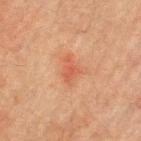Assessment:
This lesion was catalogued during total-body skin photography and was not selected for biopsy.
Image and clinical context:
The lesion's longest dimension is about 3 mm. Located on the left upper arm. An algorithmic analysis of the crop reported a footprint of about 4.5 mm². It also reported an average lesion color of about L≈48 a*≈26 b*≈31 (CIELAB), a lesion–skin lightness drop of about 7, and a normalized border contrast of about 5.5. And it measured a border-irregularity rating of about 4/10 and a within-lesion color-variation index near 1.5/10. It also reported a nevus-likeness score of about 5/100. A male patient, in their mid- to late 70s. A region of skin cropped from a whole-body photographic capture, roughly 15 mm wide. The tile uses cross-polarized illumination.